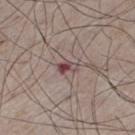Cropped from a total-body skin-imaging series; the visible field is about 15 mm.
From the left thigh.
The patient is a male in their mid- to late 70s.
This is a white-light tile.